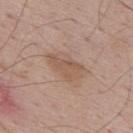Captured during whole-body skin photography for melanoma surveillance; the lesion was not biopsied.
The tile uses white-light illumination.
This image is a 15 mm lesion crop taken from a total-body photograph.
Automated tile analysis of the lesion measured a mean CIELAB color near L≈55 a*≈17 b*≈28, roughly 7 lightness units darker than nearby skin, and a normalized lesion–skin contrast near 5.5. The software also gave a border-irregularity index near 2.5/10, a color-variation rating of about 3/10, and radial color variation of about 1.
A male patient aged 68–72.
The recorded lesion diameter is about 4.5 mm.
On the upper back.